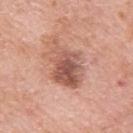Impression:
This lesion was catalogued during total-body skin photography and was not selected for biopsy.
Image and clinical context:
Automated image analysis of the tile measured a lesion area of about 15 mm², a shape eccentricity near 0.75, and a symmetry-axis asymmetry near 0.3. It also reported an average lesion color of about L≈56 a*≈24 b*≈28 (CIELAB), roughly 13 lightness units darker than nearby skin, and a normalized border contrast of about 8.5. And it measured a border-irregularity rating of about 4.5/10 and a within-lesion color-variation index near 6/10. The subject is a male roughly 80 years of age. About 6 mm across. Captured under white-light illumination. A 15 mm close-up extracted from a 3D total-body photography capture. Located on the upper back.